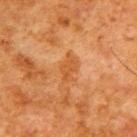Part of a total-body skin-imaging series; this lesion was reviewed on a skin check and was not flagged for biopsy. The recorded lesion diameter is about 3 mm. A region of skin cropped from a whole-body photographic capture, roughly 15 mm wide. Captured under cross-polarized illumination. A male subject aged 78 to 82. From the upper back. Automated tile analysis of the lesion measured a lesion area of about 4 mm². It also reported a lesion color around L≈42 a*≈23 b*≈37 in CIELAB and a lesion-to-skin contrast of about 6 (normalized; higher = more distinct). The software also gave a color-variation rating of about 2/10 and a peripheral color-asymmetry measure near 1. The analysis additionally found a lesion-detection confidence of about 100/100.